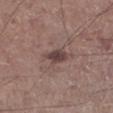Located on the left lower leg.
Longest diameter approximately 3 mm.
A lesion tile, about 15 mm wide, cut from a 3D total-body photograph.
Automated tile analysis of the lesion measured a shape eccentricity near 0.8 and a shape-asymmetry score of about 0.2 (0 = symmetric). The software also gave a lesion color around L≈41 a*≈17 b*≈18 in CIELAB, roughly 11 lightness units darker than nearby skin, and a normalized border contrast of about 9. The analysis additionally found border irregularity of about 2 on a 0–10 scale and a within-lesion color-variation index near 1.5/10. It also reported an automated nevus-likeness rating near 55 out of 100 and a detector confidence of about 100 out of 100 that the crop contains a lesion.
A male patient, in their mid-60s.
This is a white-light tile.